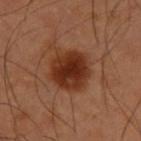  biopsy_status: not biopsied; imaged during a skin examination
  lighting: cross-polarized
  lesion_size:
    long_diameter_mm_approx: 5.0
  site: upper back
  image:
    source: total-body photography crop
    field_of_view_mm: 15
  patient:
    sex: male
    age_approx: 55